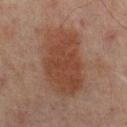Clinical impression: No biopsy was performed on this lesion — it was imaged during a full skin examination and was not determined to be concerning. Clinical summary: The lesion's longest dimension is about 8.5 mm. The lesion is on the mid back. The patient is a male in their mid- to late 60s. A close-up tile cropped from a whole-body skin photograph, about 15 mm across.